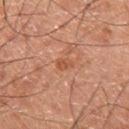{
  "biopsy_status": "not biopsied; imaged during a skin examination",
  "patient": {
    "sex": "male",
    "age_approx": 60
  },
  "image": {
    "source": "total-body photography crop",
    "field_of_view_mm": 15
  },
  "site": "right thigh"
}